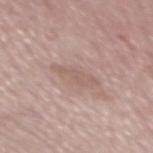This lesion was catalogued during total-body skin photography and was not selected for biopsy.